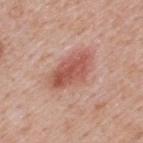Captured during whole-body skin photography for melanoma surveillance; the lesion was not biopsied.
A male patient, in their 40s.
A region of skin cropped from a whole-body photographic capture, roughly 15 mm wide.
Approximately 5.5 mm at its widest.
On the back.
This is a white-light tile.